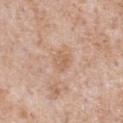<tbp_lesion>
<biopsy_status>not biopsied; imaged during a skin examination</biopsy_status>
<patient>
  <sex>male</sex>
  <age_approx>65</age_approx>
</patient>
<lesion_size>
  <long_diameter_mm_approx>2.5</long_diameter_mm_approx>
</lesion_size>
<site>chest</site>
<automated_metrics>
  <cielab_L>61</cielab_L>
  <cielab_a>19</cielab_a>
  <cielab_b>31</cielab_b>
  <vs_skin_darker_L>7.0</vs_skin_darker_L>
  <vs_skin_contrast_norm>5.0</vs_skin_contrast_norm>
</automated_metrics>
<image>
  <source>total-body photography crop</source>
  <field_of_view_mm>15</field_of_view_mm>
</image>
<lighting>white-light</lighting>
</tbp_lesion>